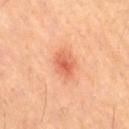Captured during whole-body skin photography for melanoma surveillance; the lesion was not biopsied.
A roughly 15 mm field-of-view crop from a total-body skin photograph.
The lesion's longest dimension is about 3.5 mm.
A female patient, approximately 40 years of age.
The lesion is on the leg.
Captured under cross-polarized illumination.